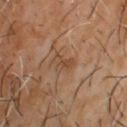No biopsy was performed on this lesion — it was imaged during a full skin examination and was not determined to be concerning.
A roughly 15 mm field-of-view crop from a total-body skin photograph.
The recorded lesion diameter is about 3 mm.
Imaged with cross-polarized lighting.
From the upper back.
A male subject aged 63 to 67.